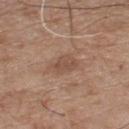Assessment: Imaged during a routine full-body skin examination; the lesion was not biopsied and no histopathology is available. Background: A 15 mm close-up extracted from a 3D total-body photography capture. A male patient aged 73–77. The lesion is located on the front of the torso.